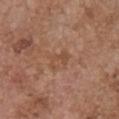Assessment:
The lesion was photographed on a routine skin check and not biopsied; there is no pathology result.
Clinical summary:
A region of skin cropped from a whole-body photographic capture, roughly 15 mm wide. A female subject, roughly 65 years of age. The lesion is on the front of the torso.